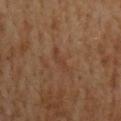Recorded during total-body skin imaging; not selected for excision or biopsy. Measured at roughly 3.5 mm in maximum diameter. This is a cross-polarized tile. A male subject in their 60s. A region of skin cropped from a whole-body photographic capture, roughly 15 mm wide. From the mid back.